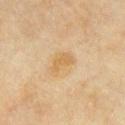Q: Was this lesion biopsied?
A: no biopsy performed (imaged during a skin exam)
Q: How was this image acquired?
A: ~15 mm crop, total-body skin-cancer survey
Q: Patient demographics?
A: male, aged approximately 60
Q: How was the tile lit?
A: cross-polarized illumination
Q: Lesion location?
A: the mid back
Q: Automated lesion metrics?
A: a lesion color around L≈63 a*≈15 b*≈41 in CIELAB, about 7 CIELAB-L* units darker than the surrounding skin, and a lesion-to-skin contrast of about 6.5 (normalized; higher = more distinct)
Q: Lesion size?
A: about 3 mm